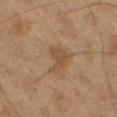Case summary:
- notes: imaged on a skin check; not biopsied
- lesion size: ~3 mm (longest diameter)
- tile lighting: cross-polarized illumination
- site: the left thigh
- subject: male, aged 73 to 77
- image-analysis metrics: a lesion color around L≈38 a*≈14 b*≈27 in CIELAB and a lesion-to-skin contrast of about 6 (normalized; higher = more distinct); a border-irregularity rating of about 4.5/10, a within-lesion color-variation index near 1.5/10, and peripheral color asymmetry of about 0.5
- image: ~15 mm tile from a whole-body skin photo